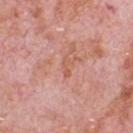Recorded during total-body skin imaging; not selected for excision or biopsy.
An algorithmic analysis of the crop reported a lesion area of about 2.5 mm², an eccentricity of roughly 0.9, and a symmetry-axis asymmetry near 0.35. The software also gave a lesion color around L≈58 a*≈27 b*≈30 in CIELAB and roughly 6 lightness units darker than nearby skin.
The lesion is on the chest.
A male patient approximately 80 years of age.
A close-up tile cropped from a whole-body skin photograph, about 15 mm across.
Longest diameter approximately 2.5 mm.